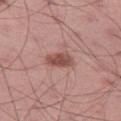No biopsy was performed on this lesion — it was imaged during a full skin examination and was not determined to be concerning.
A region of skin cropped from a whole-body photographic capture, roughly 15 mm wide.
A male subject, aged 43 to 47.
Located on the right thigh.